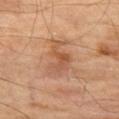The lesion was tiled from a total-body skin photograph and was not biopsied.
A close-up tile cropped from a whole-body skin photograph, about 15 mm across.
Longest diameter approximately 4.5 mm.
The lesion is located on the left thigh.
A male patient, aged around 70.